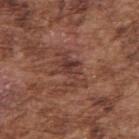Part of a total-body skin-imaging series; this lesion was reviewed on a skin check and was not flagged for biopsy.
A 15 mm close-up extracted from a 3D total-body photography capture.
A male subject, in their mid-70s.
About 5 mm across.
The lesion is located on the right upper arm.
Captured under white-light illumination.